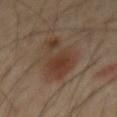Imaged during a routine full-body skin examination; the lesion was not biopsied and no histopathology is available. The lesion is on the mid back. Approximately 7.5 mm at its widest. A male patient roughly 60 years of age. The tile uses cross-polarized illumination. A region of skin cropped from a whole-body photographic capture, roughly 15 mm wide.